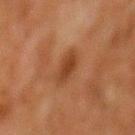workup: no biopsy performed (imaged during a skin exam) | TBP lesion metrics: a lesion area of about 5 mm² and an outline eccentricity of about 0.6 (0 = round, 1 = elongated); a lesion-detection confidence of about 100/100 | lighting: cross-polarized | lesion size: about 2.5 mm | acquisition: ~15 mm crop, total-body skin-cancer survey | body site: the mid back | subject: male, in their 80s.